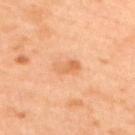{
  "biopsy_status": "not biopsied; imaged during a skin examination",
  "lesion_size": {
    "long_diameter_mm_approx": 3.0
  },
  "site": "upper back",
  "patient": {
    "sex": "male",
    "age_approx": 45
  },
  "image": {
    "source": "total-body photography crop",
    "field_of_view_mm": 15
  },
  "lighting": "cross-polarized"
}